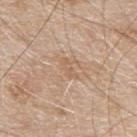Q: Who is the patient?
A: male, aged around 80
Q: Automated lesion metrics?
A: a lesion area of about 2.5 mm² and a shape eccentricity near 0.9; lesion-presence confidence of about 90/100
Q: Lesion size?
A: about 2.5 mm
Q: Illumination type?
A: white-light illumination
Q: What kind of image is this?
A: 15 mm crop, total-body photography
Q: What is the anatomic site?
A: the upper back